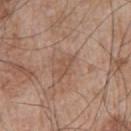Q: Was this lesion biopsied?
A: catalogued during a skin exam; not biopsied
Q: Automated lesion metrics?
A: an average lesion color of about L≈52 a*≈19 b*≈29 (CIELAB), a lesion–skin lightness drop of about 7, and a normalized border contrast of about 5; a border-irregularity index near 4/10, a within-lesion color-variation index near 1/10, and radial color variation of about 0
Q: Lesion size?
A: ~3 mm (longest diameter)
Q: What is the anatomic site?
A: the arm
Q: What are the patient's age and sex?
A: male, approximately 65 years of age
Q: Illumination type?
A: white-light illumination
Q: How was this image acquired?
A: total-body-photography crop, ~15 mm field of view